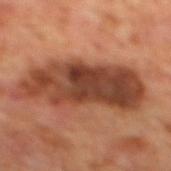Q: Was this lesion biopsied?
A: total-body-photography surveillance lesion; no biopsy
Q: How was this image acquired?
A: ~15 mm tile from a whole-body skin photo
Q: Patient demographics?
A: male, aged 68–72
Q: How large is the lesion?
A: ≈11 mm
Q: Where on the body is the lesion?
A: the mid back
Q: What lighting was used for the tile?
A: cross-polarized